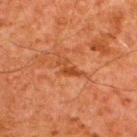| key | value |
|---|---|
| workup | imaged on a skin check; not biopsied |
| lighting | cross-polarized |
| image-analysis metrics | an area of roughly 3 mm² and a symmetry-axis asymmetry near 0.45; roughly 7 lightness units darker than nearby skin and a lesion-to-skin contrast of about 7 (normalized; higher = more distinct); border irregularity of about 6.5 on a 0–10 scale, a within-lesion color-variation index near 0/10, and a peripheral color-asymmetry measure near 0 |
| subject | male, aged 58–62 |
| image | total-body-photography crop, ~15 mm field of view |
| site | the upper back |
| diameter | ~3.5 mm (longest diameter) |